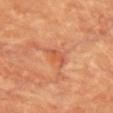| feature | finding |
|---|---|
| notes | imaged on a skin check; not biopsied |